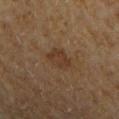Context: A lesion tile, about 15 mm wide, cut from a 3D total-body photograph. This is a cross-polarized tile. The recorded lesion diameter is about 3.5 mm. On the left upper arm. The patient is a male aged 63 to 67.